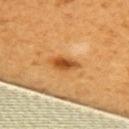The lesion was tiled from a total-body skin photograph and was not biopsied. A roughly 15 mm field-of-view crop from a total-body skin photograph. Imaged with cross-polarized lighting. Approximately 3.5 mm at its widest. The patient is a female in their mid- to late 50s. The lesion is on the upper back.